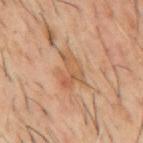The lesion was tiled from a total-body skin photograph and was not biopsied. A male patient, approximately 60 years of age. The lesion is on the mid back. A close-up tile cropped from a whole-body skin photograph, about 15 mm across.